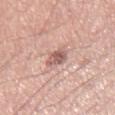Q: Was this lesion biopsied?
A: total-body-photography surveillance lesion; no biopsy
Q: What did automated image analysis measure?
A: an eccentricity of roughly 0.65 and a shape-asymmetry score of about 0.25 (0 = symmetric); a mean CIELAB color near L≈58 a*≈21 b*≈24, a lesion–skin lightness drop of about 13, and a normalized lesion–skin contrast near 8; an automated nevus-likeness rating near 5 out of 100 and lesion-presence confidence of about 100/100
Q: Patient demographics?
A: male, approximately 70 years of age
Q: What is the anatomic site?
A: the right thigh
Q: What is the lesion's diameter?
A: ≈2.5 mm
Q: What is the imaging modality?
A: ~15 mm crop, total-body skin-cancer survey
Q: Illumination type?
A: white-light